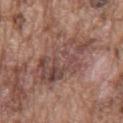Imaged during a routine full-body skin examination; the lesion was not biopsied and no histopathology is available. The lesion is on the chest. The patient is a male aged 73 to 77. A lesion tile, about 15 mm wide, cut from a 3D total-body photograph. The tile uses white-light illumination.